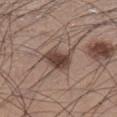Q: Was this lesion biopsied?
A: total-body-photography surveillance lesion; no biopsy
Q: What is the anatomic site?
A: the left lower leg
Q: How large is the lesion?
A: ~4 mm (longest diameter)
Q: Illumination type?
A: white-light
Q: What are the patient's age and sex?
A: male, aged 33–37
Q: What did automated image analysis measure?
A: a footprint of about 8.5 mm² and a symmetry-axis asymmetry near 0.3; a lesion-detection confidence of about 100/100
Q: What kind of image is this?
A: total-body-photography crop, ~15 mm field of view